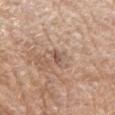Imaged during a routine full-body skin examination; the lesion was not biopsied and no histopathology is available.
From the right upper arm.
Cropped from a whole-body photographic skin survey; the tile spans about 15 mm.
The subject is a male aged approximately 70.